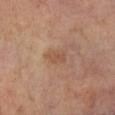biopsy status = total-body-photography surveillance lesion; no biopsy | TBP lesion metrics = a footprint of about 3.5 mm², a shape eccentricity near 0.75, and two-axis asymmetry of about 0.3; an average lesion color of about L≈50 a*≈19 b*≈30 (CIELAB), a lesion–skin lightness drop of about 7, and a normalized border contrast of about 5.5; a border-irregularity rating of about 2.5/10 and peripheral color asymmetry of about 0.5 | anatomic site = the right lower leg | patient = male, in their 70s | acquisition = 15 mm crop, total-body photography.